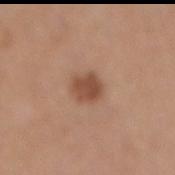Assessment: Part of a total-body skin-imaging series; this lesion was reviewed on a skin check and was not flagged for biopsy. Image and clinical context: The subject is a male about 70 years old. This image is a 15 mm lesion crop taken from a total-body photograph. On the mid back. The lesion-visualizer software estimated a footprint of about 6.5 mm², a shape eccentricity near 0.55, and a symmetry-axis asymmetry near 0.25. The software also gave lesion-presence confidence of about 100/100. The lesion's longest dimension is about 3 mm. Captured under white-light illumination.